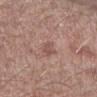Notes:
• notes — total-body-photography surveillance lesion; no biopsy
• acquisition — total-body-photography crop, ~15 mm field of view
• patient — male, about 70 years old
• lesion size — ~3 mm (longest diameter)
• body site — the arm